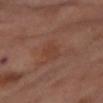{"site": "abdomen", "image": {"source": "total-body photography crop", "field_of_view_mm": 15}, "patient": {"sex": "female", "age_approx": 55}, "automated_metrics": {"area_mm2_approx": 6.0, "eccentricity": 0.8, "shape_asymmetry": 0.2, "nevus_likeness_0_100": 0, "lesion_detection_confidence_0_100": 100}, "lighting": "cross-polarized", "lesion_size": {"long_diameter_mm_approx": 3.5}}